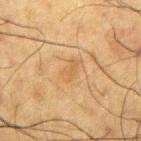Clinical impression:
No biopsy was performed on this lesion — it was imaged during a full skin examination and was not determined to be concerning.
Clinical summary:
The lesion is located on the right upper arm. A lesion tile, about 15 mm wide, cut from a 3D total-body photograph. Automated image analysis of the tile measured an average lesion color of about L≈47 a*≈16 b*≈34 (CIELAB), a lesion–skin lightness drop of about 5, and a normalized lesion–skin contrast near 4.5. And it measured a border-irregularity index near 3/10 and peripheral color asymmetry of about 0. The subject is a male aged approximately 60.